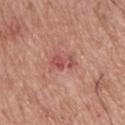Background:
The lesion is located on the back. Measured at roughly 3 mm in maximum diameter. A male subject aged 63 to 67. The tile uses white-light illumination. This image is a 15 mm lesion crop taken from a total-body photograph.